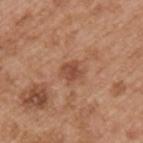Approximately 2.5 mm at its widest. The lesion-visualizer software estimated a footprint of about 4.5 mm² and an eccentricity of roughly 0.5. And it measured roughly 9 lightness units darker than nearby skin and a normalized border contrast of about 7. The analysis additionally found border irregularity of about 2.5 on a 0–10 scale. The software also gave a classifier nevus-likeness of about 5/100 and lesion-presence confidence of about 100/100. On the left upper arm. A male patient, in their mid- to late 50s. A 15 mm close-up tile from a total-body photography series done for melanoma screening.